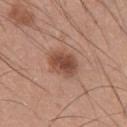biopsy_status: not biopsied; imaged during a skin examination
image:
  source: total-body photography crop
  field_of_view_mm: 15
patient:
  sex: male
  age_approx: 25
site: chest